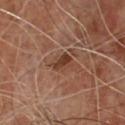{"biopsy_status": "not biopsied; imaged during a skin examination", "patient": {"sex": "male", "age_approx": 65}, "lesion_size": {"long_diameter_mm_approx": 3.0}, "lighting": "cross-polarized", "image": {"source": "total-body photography crop", "field_of_view_mm": 15}}